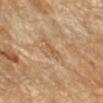workup: catalogued during a skin exam; not biopsied | location: the arm | acquisition: ~15 mm tile from a whole-body skin photo | size: about 2.5 mm | patient: female, about 70 years old | illumination: cross-polarized.